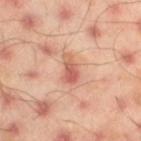| feature | finding |
|---|---|
| notes | no biopsy performed (imaged during a skin exam) |
| illumination | cross-polarized illumination |
| patient | male, approximately 45 years of age |
| diameter | ≈3.5 mm |
| image source | ~15 mm crop, total-body skin-cancer survey |
| body site | the leg |